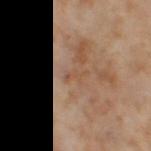Recorded during total-body skin imaging; not selected for excision or biopsy.
The subject is a female approximately 55 years of age.
A 15 mm crop from a total-body photograph taken for skin-cancer surveillance.
From the leg.